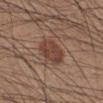{
  "biopsy_status": "not biopsied; imaged during a skin examination",
  "lighting": "white-light",
  "image": {
    "source": "total-body photography crop",
    "field_of_view_mm": 15
  },
  "site": "right lower leg",
  "lesion_size": {
    "long_diameter_mm_approx": 4.5
  },
  "patient": {
    "sex": "male",
    "age_approx": 35
  }
}